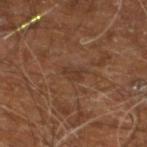Clinical summary:
The lesion-visualizer software estimated about 5 CIELAB-L* units darker than the surrounding skin and a normalized lesion–skin contrast near 5. The tile uses cross-polarized illumination. A male subject about 60 years old. Longest diameter approximately 2.5 mm. A region of skin cropped from a whole-body photographic capture, roughly 15 mm wide. The lesion is located on the leg.